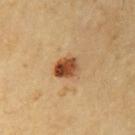The lesion was photographed on a routine skin check and not biopsied; there is no pathology result.
Located on the right upper arm.
This is a cross-polarized tile.
A region of skin cropped from a whole-body photographic capture, roughly 15 mm wide.
Measured at roughly 3 mm in maximum diameter.
The lesion-visualizer software estimated a border-irregularity index near 1.5/10, internal color variation of about 7 on a 0–10 scale, and peripheral color asymmetry of about 2.5.
A male subject, aged 68 to 72.